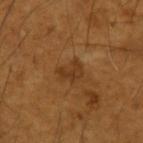  biopsy_status: not biopsied; imaged during a skin examination
  lighting: cross-polarized
  lesion_size:
    long_diameter_mm_approx: 3.0
  patient:
    sex: male
    age_approx: 65
  site: left forearm
  image:
    source: total-body photography crop
    field_of_view_mm: 15